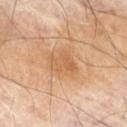No biopsy was performed on this lesion — it was imaged during a full skin examination and was not determined to be concerning.
From the right thigh.
Cropped from a total-body skin-imaging series; the visible field is about 15 mm.
Automated image analysis of the tile measured an area of roughly 7 mm², an eccentricity of roughly 0.7, and two-axis asymmetry of about 0.3. And it measured a lesion color around L≈59 a*≈21 b*≈38 in CIELAB. It also reported a border-irregularity rating of about 3/10, internal color variation of about 3 on a 0–10 scale, and radial color variation of about 1. It also reported an automated nevus-likeness rating near 5 out of 100 and a lesion-detection confidence of about 100/100.
Imaged with cross-polarized lighting.
A male subject aged approximately 70.